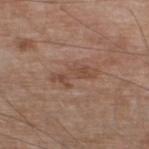<record>
<biopsy_status>not biopsied; imaged during a skin examination</biopsy_status>
<image>
  <source>total-body photography crop</source>
  <field_of_view_mm>15</field_of_view_mm>
</image>
<patient>
  <sex>male</sex>
  <age_approx>80</age_approx>
</patient>
<site>leg</site>
<automated_metrics>
  <nevus_likeness_0_100>0</nevus_likeness_0_100>
  <lesion_detection_confidence_0_100>100</lesion_detection_confidence_0_100>
</automated_metrics>
</record>